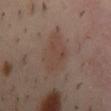The lesion was photographed on a routine skin check and not biopsied; there is no pathology result. This is a cross-polarized tile. Automated image analysis of the tile measured an area of roughly 13 mm² and a shape-asymmetry score of about 0.2 (0 = symmetric). It also reported a mean CIELAB color near L≈41 a*≈16 b*≈22 and a normalized lesion–skin contrast near 5. The software also gave a color-variation rating of about 2.5/10 and peripheral color asymmetry of about 1. And it measured a classifier nevus-likeness of about 10/100 and a detector confidence of about 100 out of 100 that the crop contains a lesion. A male patient, aged 38 to 42. Longest diameter approximately 6 mm. A 15 mm close-up extracted from a 3D total-body photography capture. The lesion is located on the mid back.